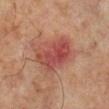Q: Was this lesion biopsied?
A: imaged on a skin check; not biopsied
Q: What is the lesion's diameter?
A: ≈5.5 mm
Q: Lesion location?
A: the right lower leg
Q: Automated lesion metrics?
A: an area of roughly 17 mm²; border irregularity of about 3 on a 0–10 scale, a color-variation rating of about 5/10, and peripheral color asymmetry of about 1.5; an automated nevus-likeness rating near 10 out of 100 and a lesion-detection confidence of about 100/100
Q: Illumination type?
A: cross-polarized
Q: Patient demographics?
A: male, about 65 years old
Q: How was this image acquired?
A: ~15 mm crop, total-body skin-cancer survey